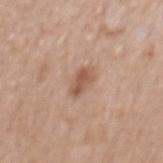Q: Is there a histopathology result?
A: catalogued during a skin exam; not biopsied
Q: How was the tile lit?
A: white-light illumination
Q: Where on the body is the lesion?
A: the mid back
Q: How large is the lesion?
A: ≈3 mm
Q: Patient demographics?
A: male, approximately 65 years of age
Q: How was this image acquired?
A: 15 mm crop, total-body photography